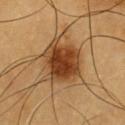  site: chest
  patient:
    sex: male
    age_approx: 60
  image:
    source: total-body photography crop
    field_of_view_mm: 15